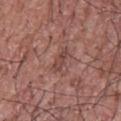Impression:
No biopsy was performed on this lesion — it was imaged during a full skin examination and was not determined to be concerning.
Image and clinical context:
A close-up tile cropped from a whole-body skin photograph, about 15 mm across. The recorded lesion diameter is about 3.5 mm. A male subject roughly 70 years of age. The lesion is on the upper back. This is a white-light tile.